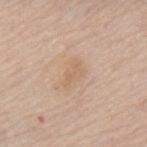Clinical impression:
Captured during whole-body skin photography for melanoma surveillance; the lesion was not biopsied.
Context:
An algorithmic analysis of the crop reported a lesion color around L≈63 a*≈16 b*≈32 in CIELAB, roughly 6 lightness units darker than nearby skin, and a normalized border contrast of about 4.5. The software also gave border irregularity of about 4 on a 0–10 scale, a within-lesion color-variation index near 1.5/10, and peripheral color asymmetry of about 0.5. The analysis additionally found an automated nevus-likeness rating near 0 out of 100. The recorded lesion diameter is about 3.5 mm. Captured under white-light illumination. A male patient aged 83 or older. This image is a 15 mm lesion crop taken from a total-body photograph. On the mid back.